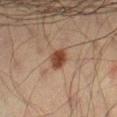This is a cross-polarized tile. A male patient about 70 years old. Automated tile analysis of the lesion measured a shape eccentricity near 0.65 and a shape-asymmetry score of about 0.25 (0 = symmetric). On the right thigh. Longest diameter approximately 2.5 mm. A region of skin cropped from a whole-body photographic capture, roughly 15 mm wide.